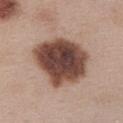Impression: No biopsy was performed on this lesion — it was imaged during a full skin examination and was not determined to be concerning. Clinical summary: A female subject aged 38–42. Captured under white-light illumination. Located on the abdomen. Cropped from a whole-body photographic skin survey; the tile spans about 15 mm.